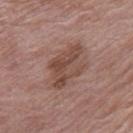Part of a total-body skin-imaging series; this lesion was reviewed on a skin check and was not flagged for biopsy. A female subject, roughly 70 years of age. The total-body-photography lesion software estimated an area of roughly 13 mm², an eccentricity of roughly 0.8, and a symmetry-axis asymmetry near 0.25. The software also gave a classifier nevus-likeness of about 0/100 and a detector confidence of about 100 out of 100 that the crop contains a lesion. Captured under white-light illumination. On the right thigh. A lesion tile, about 15 mm wide, cut from a 3D total-body photograph.